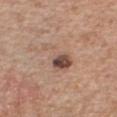Assessment: Recorded during total-body skin imaging; not selected for excision or biopsy. Clinical summary: From the chest. Captured under white-light illumination. The subject is a male about 65 years old. The recorded lesion diameter is about 4.5 mm. Cropped from a total-body skin-imaging series; the visible field is about 15 mm.